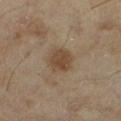Clinical impression:
Recorded during total-body skin imaging; not selected for excision or biopsy.
Clinical summary:
The recorded lesion diameter is about 3.5 mm. The lesion is on the leg. A female patient roughly 60 years of age. This image is a 15 mm lesion crop taken from a total-body photograph. Automated tile analysis of the lesion measured about 8 CIELAB-L* units darker than the surrounding skin and a normalized lesion–skin contrast near 7.5. Imaged with cross-polarized lighting.